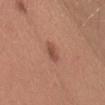notes: total-body-photography surveillance lesion; no biopsy
subject: male, in their 30s
anatomic site: the chest
image source: ~15 mm tile from a whole-body skin photo
size: ~3 mm (longest diameter)
tile lighting: white-light illumination